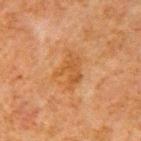notes: total-body-photography surveillance lesion; no biopsy | subject: male, about 80 years old | image source: total-body-photography crop, ~15 mm field of view | lesion diameter: about 4 mm | site: the right upper arm.